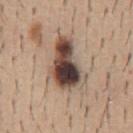Notes:
- notes · imaged on a skin check; not biopsied
- automated metrics · a footprint of about 19 mm² and an outline eccentricity of about 0.85 (0 = round, 1 = elongated); roughly 20 lightness units darker than nearby skin and a normalized lesion–skin contrast near 14.5; an automated nevus-likeness rating near 75 out of 100 and a lesion-detection confidence of about 100/100
- lesion diameter · ~6.5 mm (longest diameter)
- tile lighting · white-light
- body site · the abdomen
- patient · male, aged around 40
- acquisition · ~15 mm crop, total-body skin-cancer survey